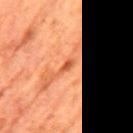workup=catalogued during a skin exam; not biopsied | acquisition=total-body-photography crop, ~15 mm field of view | subject=male, aged 63–67 | lesion size=~2.5 mm (longest diameter) | TBP lesion metrics=a lesion area of about 2 mm², a shape eccentricity near 0.9, and a symmetry-axis asymmetry near 0.25; a detector confidence of about 100 out of 100 that the crop contains a lesion | tile lighting=cross-polarized illumination | anatomic site=the mid back.